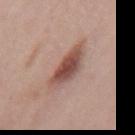| field | value |
|---|---|
| subject | male, aged 68–72 |
| acquisition | ~15 mm tile from a whole-body skin photo |
| site | the mid back |
| lesion diameter | ~5.5 mm (longest diameter) |
| tile lighting | white-light |